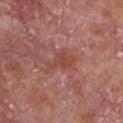- biopsy status: catalogued during a skin exam; not biopsied
- imaging modality: total-body-photography crop, ~15 mm field of view
- subject: male, in their mid-60s
- site: the front of the torso